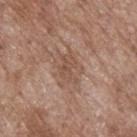The patient is a male aged around 70. This image is a 15 mm lesion crop taken from a total-body photograph. Located on the upper back. The total-body-photography lesion software estimated an automated nevus-likeness rating near 0 out of 100 and a detector confidence of about 95 out of 100 that the crop contains a lesion. This is a white-light tile.